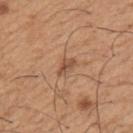Impression: Imaged during a routine full-body skin examination; the lesion was not biopsied and no histopathology is available. Context: On the left upper arm. A roughly 15 mm field-of-view crop from a total-body skin photograph. A male patient, aged 63 to 67. Longest diameter approximately 2.5 mm. Captured under white-light illumination.